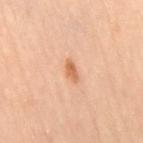  biopsy_status: not biopsied; imaged during a skin examination
  patient:
    sex: female
    age_approx: 40
  site: left leg
  lighting: cross-polarized
  lesion_size:
    long_diameter_mm_approx: 2.5
  image:
    source: total-body photography crop
    field_of_view_mm: 15